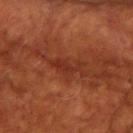| field | value |
|---|---|
| biopsy status | catalogued during a skin exam; not biopsied |
| image | 15 mm crop, total-body photography |
| subject | female, approximately 80 years of age |
| body site | the upper back |